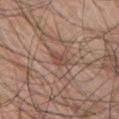Captured during whole-body skin photography for melanoma surveillance; the lesion was not biopsied. This image is a 15 mm lesion crop taken from a total-body photograph. The patient is a male in their 70s. The lesion-visualizer software estimated an outline eccentricity of about 0.85 (0 = round, 1 = elongated) and a symmetry-axis asymmetry near 0.35. And it measured a mean CIELAB color near L≈48 a*≈17 b*≈27 and a lesion–skin lightness drop of about 7. The software also gave a classifier nevus-likeness of about 0/100. This is a white-light tile. The lesion is located on the chest.